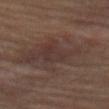follow-up: catalogued during a skin exam; not biopsied
diameter: ≈9.5 mm
image: total-body-photography crop, ~15 mm field of view
lighting: cross-polarized
location: the abdomen
patient: male, aged 53 to 57